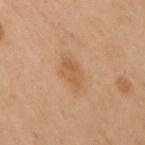Impression: The lesion was tiled from a total-body skin photograph and was not biopsied. Context: The patient is a female aged approximately 60. On the upper back. A 15 mm crop from a total-body photograph taken for skin-cancer surveillance.